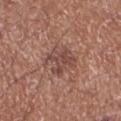biopsy status — total-body-photography surveillance lesion; no biopsy
tile lighting — white-light illumination
location — the left lower leg
diameter — about 4 mm
subject — male, aged 68 to 72
image source — ~15 mm tile from a whole-body skin photo